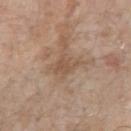Clinical impression: This lesion was catalogued during total-body skin photography and was not selected for biopsy. Image and clinical context: This is a white-light tile. Automated image analysis of the tile measured an automated nevus-likeness rating near 0 out of 100 and lesion-presence confidence of about 85/100. This image is a 15 mm lesion crop taken from a total-body photograph. Located on the right upper arm. A female subject, about 85 years old or older. The lesion's longest dimension is about 3.5 mm.